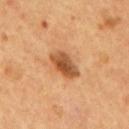Impression: The lesion was tiled from a total-body skin photograph and was not biopsied. Background: Longest diameter approximately 4 mm. On the mid back. A male patient aged approximately 55. Cropped from a whole-body photographic skin survey; the tile spans about 15 mm.